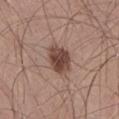Imaged during a routine full-body skin examination; the lesion was not biopsied and no histopathology is available.
About 3.5 mm across.
Imaged with white-light lighting.
Located on the right thigh.
A region of skin cropped from a whole-body photographic capture, roughly 15 mm wide.
The patient is a male in their mid-20s.